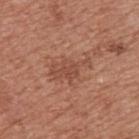Recorded during total-body skin imaging; not selected for excision or biopsy. A region of skin cropped from a whole-body photographic capture, roughly 15 mm wide. From the upper back. A male subject, aged around 55.